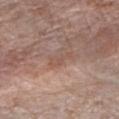Q: Was this lesion biopsied?
A: imaged on a skin check; not biopsied
Q: Who is the patient?
A: female, roughly 65 years of age
Q: Lesion location?
A: the right forearm
Q: What is the imaging modality?
A: ~15 mm crop, total-body skin-cancer survey
Q: What did automated image analysis measure?
A: border irregularity of about 6.5 on a 0–10 scale, a within-lesion color-variation index near 0/10, and peripheral color asymmetry of about 0
Q: How large is the lesion?
A: ~3.5 mm (longest diameter)
Q: How was the tile lit?
A: white-light